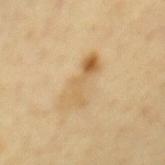Q: Was a biopsy performed?
A: no biopsy performed (imaged during a skin exam)